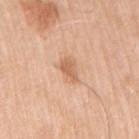Notes:
• notes · no biopsy performed (imaged during a skin exam)
• lesion diameter · ~2.5 mm (longest diameter)
• subject · male, approximately 80 years of age
• tile lighting · white-light
• imaging modality · total-body-photography crop, ~15 mm field of view
• site · the right upper arm